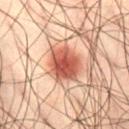| feature | finding |
|---|---|
| follow-up | no biopsy performed (imaged during a skin exam) |
| lesion diameter | ~4.5 mm (longest diameter) |
| acquisition | ~15 mm tile from a whole-body skin photo |
| subject | male, about 50 years old |
| site | the abdomen |
| illumination | cross-polarized illumination |